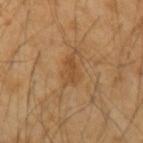Assessment: The lesion was photographed on a routine skin check and not biopsied; there is no pathology result. Background: On the left upper arm. Cropped from a total-body skin-imaging series; the visible field is about 15 mm. This is a cross-polarized tile. Automated image analysis of the tile measured a shape-asymmetry score of about 0.35 (0 = symmetric). The software also gave a lesion color around L≈48 a*≈20 b*≈37 in CIELAB and a normalized border contrast of about 5.5. And it measured a nevus-likeness score of about 10/100. A male patient in their 50s.